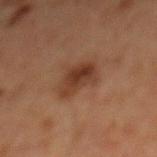Captured during whole-body skin photography for melanoma surveillance; the lesion was not biopsied. The subject is a male aged 58–62. A 15 mm crop from a total-body photograph taken for skin-cancer surveillance. The lesion is on the chest.